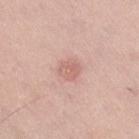Captured during whole-body skin photography for melanoma surveillance; the lesion was not biopsied. Captured under white-light illumination. Located on the left thigh. A female subject aged 58 to 62. An algorithmic analysis of the crop reported an average lesion color of about L≈64 a*≈23 b*≈25 (CIELAB), a lesion–skin lightness drop of about 8, and a normalized lesion–skin contrast near 5. And it measured lesion-presence confidence of about 100/100. Measured at roughly 2.5 mm in maximum diameter. A roughly 15 mm field-of-view crop from a total-body skin photograph.